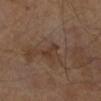| field | value |
|---|---|
| notes | total-body-photography surveillance lesion; no biopsy |
| image | ~15 mm tile from a whole-body skin photo |
| subject | male, roughly 65 years of age |